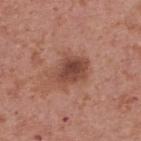Assessment:
The lesion was photographed on a routine skin check and not biopsied; there is no pathology result.
Clinical summary:
A male subject roughly 70 years of age. From the back. A 15 mm crop from a total-body photograph taken for skin-cancer surveillance. This is a white-light tile.